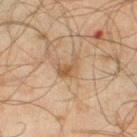workup — catalogued during a skin exam; not biopsied | imaging modality — total-body-photography crop, ~15 mm field of view | body site — the left lower leg | tile lighting — cross-polarized | subject — male, aged approximately 45 | image-analysis metrics — internal color variation of about 1.5 on a 0–10 scale and radial color variation of about 0.5; an automated nevus-likeness rating near 35 out of 100 | lesion size — ≈3 mm.